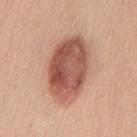Assessment: Part of a total-body skin-imaging series; this lesion was reviewed on a skin check and was not flagged for biopsy. Image and clinical context: Automated image analysis of the tile measured an area of roughly 29 mm², a shape eccentricity near 0.8, and two-axis asymmetry of about 0.1. The analysis additionally found a classifier nevus-likeness of about 90/100 and a lesion-detection confidence of about 100/100. The tile uses white-light illumination. A female subject, approximately 40 years of age. Cropped from a total-body skin-imaging series; the visible field is about 15 mm. The lesion is located on the front of the torso. About 8 mm across.